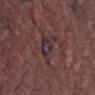Clinical impression: The lesion was tiled from a total-body skin photograph and was not biopsied. Image and clinical context: A male subject, aged approximately 55. A 15 mm close-up tile from a total-body photography series done for melanoma screening. Automated image analysis of the tile measured a classifier nevus-likeness of about 0/100. The recorded lesion diameter is about 5 mm. The lesion is on the left thigh. Imaged with white-light lighting.